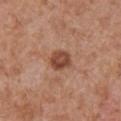Captured during whole-body skin photography for melanoma surveillance; the lesion was not biopsied.
On the chest.
The tile uses white-light illumination.
A male patient, in their mid-60s.
A lesion tile, about 15 mm wide, cut from a 3D total-body photograph.